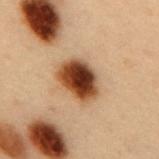Q: Is there a histopathology result?
A: imaged on a skin check; not biopsied
Q: What lighting was used for the tile?
A: cross-polarized illumination
Q: Who is the patient?
A: male, aged 53 to 57
Q: What is the anatomic site?
A: the back
Q: What is the lesion's diameter?
A: ≈4.5 mm
Q: How was this image acquired?
A: 15 mm crop, total-body photography